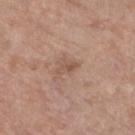A lesion tile, about 15 mm wide, cut from a 3D total-body photograph. Located on the left lower leg. This is a white-light tile. About 2.5 mm across. A female patient roughly 85 years of age or older. Automated image analysis of the tile measured a lesion color around L≈53 a*≈19 b*≈28 in CIELAB, a lesion–skin lightness drop of about 8, and a normalized border contrast of about 6. The analysis additionally found an automated nevus-likeness rating near 0 out of 100 and a lesion-detection confidence of about 100/100.